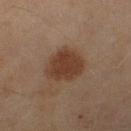Case summary:
* notes · imaged on a skin check; not biopsied
* tile lighting · cross-polarized illumination
* location · the right lower leg
* imaging modality · ~15 mm crop, total-body skin-cancer survey
* automated lesion analysis · an eccentricity of roughly 0.5; an average lesion color of about L≈35 a*≈17 b*≈26 (CIELAB), a lesion–skin lightness drop of about 9, and a lesion-to-skin contrast of about 9 (normalized; higher = more distinct); a classifier nevus-likeness of about 100/100 and a lesion-detection confidence of about 100/100
* diameter · about 4.5 mm
* subject · female, aged 58–62